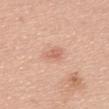Clinical impression: Part of a total-body skin-imaging series; this lesion was reviewed on a skin check and was not flagged for biopsy. Background: Automated image analysis of the tile measured a lesion area of about 3 mm² and a symmetry-axis asymmetry near 0.2. The software also gave a border-irregularity index near 2/10, a within-lesion color-variation index near 1.5/10, and peripheral color asymmetry of about 0.5. Captured under white-light illumination. The lesion's longest dimension is about 2.5 mm. On the upper back. A female patient aged 58–62. A 15 mm crop from a total-body photograph taken for skin-cancer surveillance.